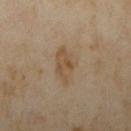Assessment:
Imaged during a routine full-body skin examination; the lesion was not biopsied and no histopathology is available.
Context:
On the arm. Imaged with cross-polarized lighting. Measured at roughly 4.5 mm in maximum diameter. A female patient aged 33–37. A region of skin cropped from a whole-body photographic capture, roughly 15 mm wide.